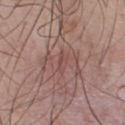Captured during whole-body skin photography for melanoma surveillance; the lesion was not biopsied.
A roughly 15 mm field-of-view crop from a total-body skin photograph.
The lesion is located on the upper back.
The total-body-photography lesion software estimated an area of roughly 2 mm², a shape eccentricity near 0.9, and a symmetry-axis asymmetry near 0.4. The software also gave an average lesion color of about L≈48 a*≈21 b*≈22 (CIELAB), about 6 CIELAB-L* units darker than the surrounding skin, and a lesion-to-skin contrast of about 4.5 (normalized; higher = more distinct). The analysis additionally found border irregularity of about 4 on a 0–10 scale and a within-lesion color-variation index near 0/10. And it measured a nevus-likeness score of about 0/100.
The subject is a male aged 43–47.
The recorded lesion diameter is about 2.5 mm.